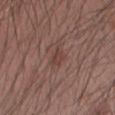The lesion was tiled from a total-body skin photograph and was not biopsied.
A male patient, in their mid- to late 40s.
The lesion is located on the left forearm.
A 15 mm crop from a total-body photograph taken for skin-cancer surveillance.